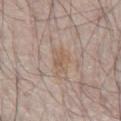follow-up = imaged on a skin check; not biopsied
patient = male, aged 53–57
location = the chest
diameter = ≈3 mm
lighting = white-light
acquisition = total-body-photography crop, ~15 mm field of view